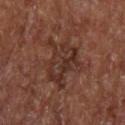Imaged during a routine full-body skin examination; the lesion was not biopsied and no histopathology is available.
The subject is a male aged approximately 55.
Longest diameter approximately 6 mm.
On the upper back.
Automated tile analysis of the lesion measured a nevus-likeness score of about 0/100 and lesion-presence confidence of about 95/100.
This image is a 15 mm lesion crop taken from a total-body photograph.